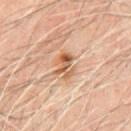This lesion was catalogued during total-body skin photography and was not selected for biopsy.
On the chest.
Measured at roughly 3 mm in maximum diameter.
A lesion tile, about 15 mm wide, cut from a 3D total-body photograph.
The total-body-photography lesion software estimated a footprint of about 5.5 mm² and an eccentricity of roughly 0.7. And it measured a lesion color around L≈58 a*≈22 b*≈35 in CIELAB and a lesion-to-skin contrast of about 8 (normalized; higher = more distinct). It also reported an automated nevus-likeness rating near 10 out of 100 and lesion-presence confidence of about 100/100.
A male patient, in their mid-60s.
Imaged with cross-polarized lighting.